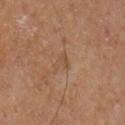The lesion was photographed on a routine skin check and not biopsied; there is no pathology result. A male subject in their mid-60s. Automated tile analysis of the lesion measured a lesion area of about 3 mm², an eccentricity of roughly 0.75, and a shape-asymmetry score of about 0.45 (0 = symmetric). The analysis additionally found about 5 CIELAB-L* units darker than the surrounding skin and a normalized border contrast of about 4.5. And it measured a border-irregularity rating of about 5/10, a color-variation rating of about 1/10, and a peripheral color-asymmetry measure near 0. The lesion's longest dimension is about 3 mm. Cropped from a total-body skin-imaging series; the visible field is about 15 mm. Located on the left upper arm. Captured under cross-polarized illumination.